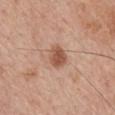Background:
A male subject, aged 53–57. A close-up tile cropped from a whole-body skin photograph, about 15 mm across. About 2.5 mm across. Imaged with white-light lighting. The lesion-visualizer software estimated an area of roughly 5.5 mm², a shape eccentricity near 0.1, and a symmetry-axis asymmetry near 0.15. The analysis additionally found a lesion color around L≈54 a*≈22 b*≈31 in CIELAB, about 12 CIELAB-L* units darker than the surrounding skin, and a lesion-to-skin contrast of about 8.5 (normalized; higher = more distinct). The software also gave a border-irregularity rating of about 1.5/10 and a within-lesion color-variation index near 2.5/10. It also reported a detector confidence of about 100 out of 100 that the crop contains a lesion. The lesion is located on the mid back.